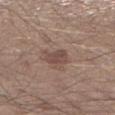The tile uses white-light illumination. The lesion's longest dimension is about 3 mm. Located on the right lower leg. A male subject aged around 30. A 15 mm close-up tile from a total-body photography series done for melanoma screening.